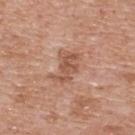notes: catalogued during a skin exam; not biopsied.